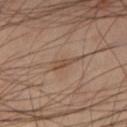Imaged with cross-polarized lighting.
A male subject aged around 50.
The lesion is located on the left lower leg.
This image is a 15 mm lesion crop taken from a total-body photograph.
Measured at roughly 3.5 mm in maximum diameter.